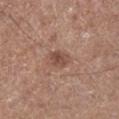Case summary:
- patient: male, roughly 60 years of age
- anatomic site: the right lower leg
- image-analysis metrics: a shape eccentricity near 0.8 and a shape-asymmetry score of about 0.3 (0 = symmetric); border irregularity of about 2.5 on a 0–10 scale, a color-variation rating of about 3/10, and radial color variation of about 1
- image source: ~15 mm crop, total-body skin-cancer survey
- lighting: white-light illumination